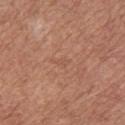This lesion was catalogued during total-body skin photography and was not selected for biopsy. Located on the upper back. Captured under white-light illumination. A lesion tile, about 15 mm wide, cut from a 3D total-body photograph. A female patient about 55 years old. The recorded lesion diameter is about 1.5 mm.